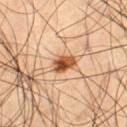biopsy status: total-body-photography surveillance lesion; no biopsy | tile lighting: cross-polarized | lesion size: about 2.5 mm | patient: male, about 55 years old | location: the leg | image: ~15 mm crop, total-body skin-cancer survey.